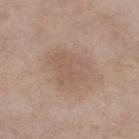Captured during whole-body skin photography for melanoma surveillance; the lesion was not biopsied.
A female subject, approximately 55 years of age.
On the right thigh.
A 15 mm close-up extracted from a 3D total-body photography capture.
The tile uses white-light illumination.
An algorithmic analysis of the crop reported an eccentricity of roughly 0.7 and a shape-asymmetry score of about 0.3 (0 = symmetric). The software also gave a lesion color around L≈57 a*≈16 b*≈26 in CIELAB, a lesion–skin lightness drop of about 6, and a lesion-to-skin contrast of about 4.5 (normalized; higher = more distinct). The software also gave a border-irregularity rating of about 3/10, a within-lesion color-variation index near 2/10, and radial color variation of about 0.5.
Longest diameter approximately 5.5 mm.